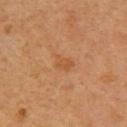No biopsy was performed on this lesion — it was imaged during a full skin examination and was not determined to be concerning.
A 15 mm crop from a total-body photograph taken for skin-cancer surveillance.
On the left upper arm.
Imaged with cross-polarized lighting.
An algorithmic analysis of the crop reported a lesion color around L≈47 a*≈21 b*≈35 in CIELAB, about 5 CIELAB-L* units darker than the surrounding skin, and a lesion-to-skin contrast of about 5 (normalized; higher = more distinct). And it measured a border-irregularity index near 3/10, internal color variation of about 1.5 on a 0–10 scale, and a peripheral color-asymmetry measure near 0.5. The software also gave a classifier nevus-likeness of about 5/100 and a lesion-detection confidence of about 100/100.
The lesion's longest dimension is about 2.5 mm.
A male subject, aged 58–62.